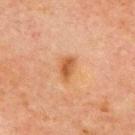Imaged during a routine full-body skin examination; the lesion was not biopsied and no histopathology is available. This image is a 15 mm lesion crop taken from a total-body photograph. From the chest. Automated image analysis of the tile measured a footprint of about 4 mm² and two-axis asymmetry of about 0.3. The analysis additionally found a border-irregularity rating of about 2.5/10 and a within-lesion color-variation index near 2.5/10. The recorded lesion diameter is about 3 mm. This is a cross-polarized tile. The subject is a male aged around 70.